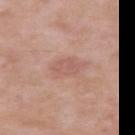Q: Was this lesion biopsied?
A: total-body-photography surveillance lesion; no biopsy
Q: Illumination type?
A: white-light illumination
Q: Lesion location?
A: the arm
Q: What are the patient's age and sex?
A: male, aged around 55
Q: What is the imaging modality?
A: ~15 mm tile from a whole-body skin photo